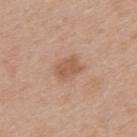Q: Was a biopsy performed?
A: catalogued during a skin exam; not biopsied
Q: How large is the lesion?
A: about 3 mm
Q: How was this image acquired?
A: ~15 mm tile from a whole-body skin photo
Q: How was the tile lit?
A: white-light illumination
Q: Lesion location?
A: the back
Q: What are the patient's age and sex?
A: male, aged approximately 30